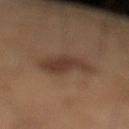{"biopsy_status": "not biopsied; imaged during a skin examination", "site": "left lower leg", "lesion_size": {"long_diameter_mm_approx": 4.5}, "patient": {"sex": "male", "age_approx": 60}, "lighting": "cross-polarized", "image": {"source": "total-body photography crop", "field_of_view_mm": 15}}